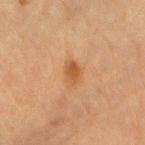Case summary:
• workup · imaged on a skin check; not biopsied
• patient · male, in their mid-80s or older
• automated lesion analysis · a lesion area of about 4 mm² and an outline eccentricity of about 0.8 (0 = round, 1 = elongated); a nevus-likeness score of about 80/100
• lighting · cross-polarized illumination
• image · 15 mm crop, total-body photography
• location · the right upper arm